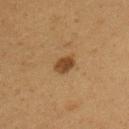No biopsy was performed on this lesion — it was imaged during a full skin examination and was not determined to be concerning.
A female subject approximately 20 years of age.
The lesion is on the left upper arm.
A close-up tile cropped from a whole-body skin photograph, about 15 mm across.
The lesion-visualizer software estimated a lesion area of about 4 mm². And it measured a border-irregularity rating of about 1/10, internal color variation of about 2 on a 0–10 scale, and a peripheral color-asymmetry measure near 1. The analysis additionally found an automated nevus-likeness rating near 95 out of 100 and lesion-presence confidence of about 100/100.
Approximately 2.5 mm at its widest.
This is a cross-polarized tile.